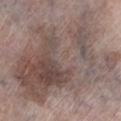No biopsy was performed on this lesion — it was imaged during a full skin examination and was not determined to be concerning.
A female subject, approximately 65 years of age.
About 14.5 mm across.
The lesion is on the left lower leg.
An algorithmic analysis of the crop reported an area of roughly 95 mm² and an eccentricity of roughly 0.75. The software also gave a mean CIELAB color near L≈50 a*≈14 b*≈20. And it measured a border-irregularity rating of about 6/10, a within-lesion color-variation index near 8.5/10, and peripheral color asymmetry of about 3.
The tile uses white-light illumination.
A 15 mm crop from a total-body photograph taken for skin-cancer surveillance.